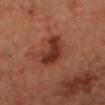This lesion was catalogued during total-body skin photography and was not selected for biopsy. About 5 mm across. The tile uses cross-polarized illumination. A male patient, in their mid-60s. A roughly 15 mm field-of-view crop from a total-body skin photograph. The lesion is located on the head or neck.